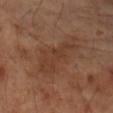The lesion was photographed on a routine skin check and not biopsied; there is no pathology result. The lesion is located on the right forearm. This is a cross-polarized tile. A close-up tile cropped from a whole-body skin photograph, about 15 mm across. A female subject aged approximately 60. The recorded lesion diameter is about 7 mm.